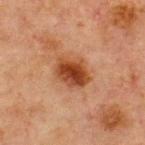Part of a total-body skin-imaging series; this lesion was reviewed on a skin check and was not flagged for biopsy. A roughly 15 mm field-of-view crop from a total-body skin photograph. A male patient, aged 63 to 67. The lesion is on the front of the torso. Automated image analysis of the tile measured a mean CIELAB color near L≈36 a*≈23 b*≈31, a lesion–skin lightness drop of about 12, and a normalized lesion–skin contrast near 10.5. Measured at roughly 4 mm in maximum diameter. This is a cross-polarized tile.